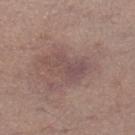Q: Was a biopsy performed?
A: imaged on a skin check; not biopsied
Q: Patient demographics?
A: female, aged around 65
Q: Where on the body is the lesion?
A: the leg
Q: What lighting was used for the tile?
A: white-light
Q: What is the imaging modality?
A: ~15 mm tile from a whole-body skin photo
Q: How large is the lesion?
A: ~5 mm (longest diameter)